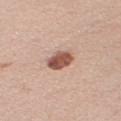Q: Is there a histopathology result?
A: imaged on a skin check; not biopsied
Q: Lesion location?
A: the right upper arm
Q: Lesion size?
A: about 3 mm
Q: How was the tile lit?
A: white-light illumination
Q: What is the imaging modality?
A: ~15 mm tile from a whole-body skin photo
Q: What are the patient's age and sex?
A: female, aged around 35
Q: Automated lesion metrics?
A: a footprint of about 6.5 mm² and a symmetry-axis asymmetry near 0.2; roughly 17 lightness units darker than nearby skin and a normalized border contrast of about 11; an automated nevus-likeness rating near 100 out of 100 and a lesion-detection confidence of about 100/100